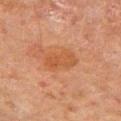Q: Was this lesion biopsied?
A: imaged on a skin check; not biopsied
Q: Who is the patient?
A: male, roughly 70 years of age
Q: How was the tile lit?
A: cross-polarized illumination
Q: What kind of image is this?
A: total-body-photography crop, ~15 mm field of view
Q: What is the lesion's diameter?
A: ≈4 mm
Q: What is the anatomic site?
A: the right upper arm